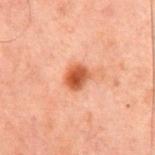workup = imaged on a skin check; not biopsied
subject = male, about 60 years old
imaging modality = ~15 mm crop, total-body skin-cancer survey
site = the chest
TBP lesion metrics = a border-irregularity index near 1.5/10 and a peripheral color-asymmetry measure near 1.5; lesion-presence confidence of about 100/100
tile lighting = cross-polarized